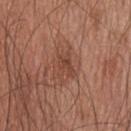Q: Is there a histopathology result?
A: imaged on a skin check; not biopsied
Q: What is the lesion's diameter?
A: ≈3.5 mm
Q: How was the tile lit?
A: white-light
Q: How was this image acquired?
A: ~15 mm tile from a whole-body skin photo
Q: Where on the body is the lesion?
A: the head or neck
Q: Who is the patient?
A: male, aged 43–47